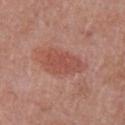Part of a total-body skin-imaging series; this lesion was reviewed on a skin check and was not flagged for biopsy.
A male subject aged approximately 50.
About 5.5 mm across.
On the chest.
The tile uses white-light illumination.
A roughly 15 mm field-of-view crop from a total-body skin photograph.